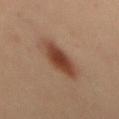Part of a total-body skin-imaging series; this lesion was reviewed on a skin check and was not flagged for biopsy. The subject is a female in their mid-30s. Cropped from a whole-body photographic skin survey; the tile spans about 15 mm. From the mid back.